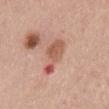image: 15 mm crop, total-body photography; body site: the abdomen; patient: female, aged 58–62; lighting: white-light illumination.